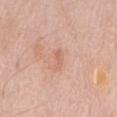Impression: Part of a total-body skin-imaging series; this lesion was reviewed on a skin check and was not flagged for biopsy. Clinical summary: A male subject approximately 60 years of age. Longest diameter approximately 2.5 mm. Captured under white-light illumination. The lesion-visualizer software estimated a footprint of about 2.5 mm², an eccentricity of roughly 0.9, and a symmetry-axis asymmetry near 0.4. The analysis additionally found a border-irregularity rating of about 4/10, a color-variation rating of about 0/10, and a peripheral color-asymmetry measure near 0. It also reported an automated nevus-likeness rating near 0 out of 100. A lesion tile, about 15 mm wide, cut from a 3D total-body photograph. The lesion is on the mid back.